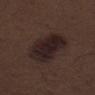notes: total-body-photography surveillance lesion; no biopsy | patient: male, in their 70s | diameter: about 5.5 mm | lighting: white-light | body site: the left thigh | image: ~15 mm crop, total-body skin-cancer survey.